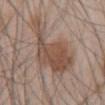workup: no biopsy performed (imaged during a skin exam)
lesion size: about 10 mm
tile lighting: white-light
patient: male, aged around 45
body site: the abdomen
image source: 15 mm crop, total-body photography
image-analysis metrics: a mean CIELAB color near L≈50 a*≈17 b*≈26, roughly 10 lightness units darker than nearby skin, and a normalized lesion–skin contrast near 7.5; an automated nevus-likeness rating near 85 out of 100 and a lesion-detection confidence of about 100/100